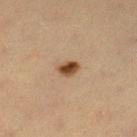Captured during whole-body skin photography for melanoma surveillance; the lesion was not biopsied. The tile uses cross-polarized illumination. Approximately 2.5 mm at its widest. A close-up tile cropped from a whole-body skin photograph, about 15 mm across. A female patient, roughly 40 years of age. Located on the left lower leg. Automated tile analysis of the lesion measured a border-irregularity index near 2/10, a within-lesion color-variation index near 4/10, and peripheral color asymmetry of about 1.5. The analysis additionally found a nevus-likeness score of about 100/100 and a lesion-detection confidence of about 100/100.